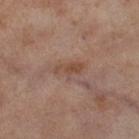No biopsy was performed on this lesion — it was imaged during a full skin examination and was not determined to be concerning.
This image is a 15 mm lesion crop taken from a total-body photograph.
From the right thigh.
A female patient roughly 55 years of age.